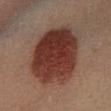Clinical impression: No biopsy was performed on this lesion — it was imaged during a full skin examination and was not determined to be concerning. Background: The lesion-visualizer software estimated a footprint of about 42 mm², a shape eccentricity near 0.6, and two-axis asymmetry of about 0.1. The software also gave a detector confidence of about 100 out of 100 that the crop contains a lesion. Cropped from a total-body skin-imaging series; the visible field is about 15 mm. The recorded lesion diameter is about 8 mm. From the leg. A male subject about 40 years old. Imaged with cross-polarized lighting.